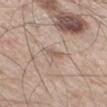{
  "biopsy_status": "not biopsied; imaged during a skin examination",
  "site": "right thigh",
  "image": {
    "source": "total-body photography crop",
    "field_of_view_mm": 15
  },
  "patient": {
    "sex": "male",
    "age_approx": 60
  },
  "lesion_size": {
    "long_diameter_mm_approx": 3.0
  }
}